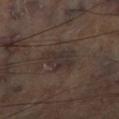Cropped from a total-body skin-imaging series; the visible field is about 15 mm.
On the right lower leg.
Automated image analysis of the tile measured a lesion color around L≈30 a*≈11 b*≈16 in CIELAB, roughly 6 lightness units darker than nearby skin, and a lesion-to-skin contrast of about 7 (normalized; higher = more distinct). The software also gave a border-irregularity index near 3/10, a within-lesion color-variation index near 3/10, and peripheral color asymmetry of about 1. And it measured a nevus-likeness score of about 0/100 and lesion-presence confidence of about 95/100.
This is a cross-polarized tile.
The lesion's longest dimension is about 4 mm.